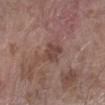Q: Was a biopsy performed?
A: imaged on a skin check; not biopsied
Q: How was the tile lit?
A: white-light illumination
Q: Where on the body is the lesion?
A: the left forearm
Q: How was this image acquired?
A: total-body-photography crop, ~15 mm field of view
Q: What are the patient's age and sex?
A: male, roughly 60 years of age